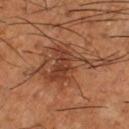Part of a total-body skin-imaging series; this lesion was reviewed on a skin check and was not flagged for biopsy. The lesion is located on the right lower leg. A region of skin cropped from a whole-body photographic capture, roughly 15 mm wide. Captured under cross-polarized illumination. The recorded lesion diameter is about 9 mm. An algorithmic analysis of the crop reported an area of roughly 25 mm², a shape eccentricity near 0.75, and a symmetry-axis asymmetry near 0.5. The analysis additionally found an average lesion color of about L≈43 a*≈23 b*≈32 (CIELAB), roughly 9 lightness units darker than nearby skin, and a normalized border contrast of about 7.5. A male patient, aged 63–67.